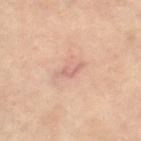| feature | finding |
|---|---|
| acquisition | 15 mm crop, total-body photography |
| automated lesion analysis | a lesion area of about 3 mm², an eccentricity of roughly 0.9, and a shape-asymmetry score of about 0.45 (0 = symmetric); roughly 8 lightness units darker than nearby skin; a border-irregularity rating of about 5/10 and radial color variation of about 0.5; an automated nevus-likeness rating near 0 out of 100 |
| lesion size | about 3 mm |
| lighting | cross-polarized |
| site | the leg |
| patient | female, approximately 60 years of age |